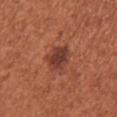Notes:
* notes — no biopsy performed (imaged during a skin exam)
* image — total-body-photography crop, ~15 mm field of view
* location — the right upper arm
* subject — female, in their mid- to late 60s
* lesion size — about 4 mm
* illumination — white-light illumination
* automated metrics — an area of roughly 9 mm² and a shape-asymmetry score of about 0.2 (0 = symmetric); a classifier nevus-likeness of about 95/100 and lesion-presence confidence of about 100/100